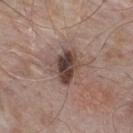Case summary:
– workup · no biopsy performed (imaged during a skin exam)
– patient · male, aged 53 to 57
– site · the upper back
– acquisition · ~15 mm crop, total-body skin-cancer survey